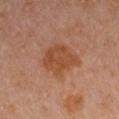biopsy status — total-body-photography surveillance lesion; no biopsy
image — ~15 mm crop, total-body skin-cancer survey
automated metrics — a lesion area of about 14 mm², a shape eccentricity near 0.5, and a shape-asymmetry score of about 0.2 (0 = symmetric); a mean CIELAB color near L≈46 a*≈23 b*≈34 and a normalized border contrast of about 7.5; border irregularity of about 2.5 on a 0–10 scale and peripheral color asymmetry of about 1
tile lighting — cross-polarized illumination
lesion size — ≈4.5 mm
site — the left upper arm
patient — female, approximately 50 years of age